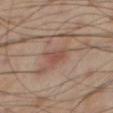Q: Was this lesion biopsied?
A: total-body-photography surveillance lesion; no biopsy